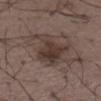A roughly 15 mm field-of-view crop from a total-body skin photograph.
On the left thigh.
A male patient in their 50s.
The total-body-photography lesion software estimated an automated nevus-likeness rating near 40 out of 100.
Approximately 5 mm at its widest.